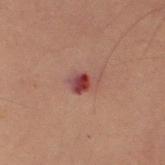- notes: no biopsy performed (imaged during a skin exam)
- image source: ~15 mm crop, total-body skin-cancer survey
- lesion size: ≈3 mm
- tile lighting: cross-polarized illumination
- body site: the right forearm
- patient: male, approximately 60 years of age